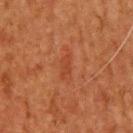Imaged during a routine full-body skin examination; the lesion was not biopsied and no histopathology is available. A 15 mm close-up tile from a total-body photography series done for melanoma screening. On the upper back. An algorithmic analysis of the crop reported an area of roughly 3 mm² and a symmetry-axis asymmetry near 0.4. And it measured a border-irregularity index near 4.5/10 and a color-variation rating of about 0.5/10. The software also gave an automated nevus-likeness rating near 0 out of 100 and a lesion-detection confidence of about 100/100. Imaged with cross-polarized lighting. Longest diameter approximately 3 mm. A male patient aged approximately 50.